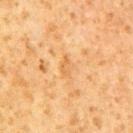Impression:
This lesion was catalogued during total-body skin photography and was not selected for biopsy.
Acquisition and patient details:
Longest diameter approximately 2.5 mm. An algorithmic analysis of the crop reported a lesion area of about 3 mm², a shape eccentricity near 0.8, and a shape-asymmetry score of about 0.35 (0 = symmetric). And it measured an automated nevus-likeness rating near 0 out of 100. Located on the right upper arm. Imaged with cross-polarized lighting. A close-up tile cropped from a whole-body skin photograph, about 15 mm across. A male subject, approximately 60 years of age.